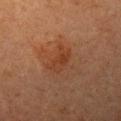Acquisition and patient details: The subject is a female roughly 60 years of age. Located on the left upper arm. A 15 mm close-up extracted from a 3D total-body photography capture.